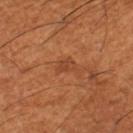| feature | finding |
|---|---|
| workup | no biopsy performed (imaged during a skin exam) |
| location | the right lower leg |
| patient | male, aged 63–67 |
| lesion diameter | ≈2.5 mm |
| automated lesion analysis | a footprint of about 4 mm², an eccentricity of roughly 0.65, and a symmetry-axis asymmetry near 0.5; a mean CIELAB color near L≈45 a*≈26 b*≈37 and about 7 CIELAB-L* units darker than the surrounding skin; a classifier nevus-likeness of about 5/100 and a detector confidence of about 100 out of 100 that the crop contains a lesion |
| image | ~15 mm crop, total-body skin-cancer survey |
| tile lighting | cross-polarized |